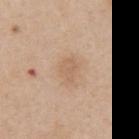Assessment: Captured during whole-body skin photography for melanoma surveillance; the lesion was not biopsied. Background: The patient is a male in their 60s. The tile uses white-light illumination. A 15 mm close-up tile from a total-body photography series done for melanoma screening. Located on the left upper arm.